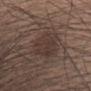Image and clinical context:
Imaged with white-light lighting. The subject is a female about 35 years old. This image is a 15 mm lesion crop taken from a total-body photograph. On the head or neck.